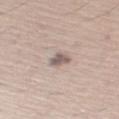Notes:
- follow-up · no biopsy performed (imaged during a skin exam)
- image · ~15 mm tile from a whole-body skin photo
- body site · the right upper arm
- lesion diameter · about 2.5 mm
- automated lesion analysis · a border-irregularity index near 3/10, internal color variation of about 3 on a 0–10 scale, and radial color variation of about 1; a classifier nevus-likeness of about 0/100 and a detector confidence of about 75 out of 100 that the crop contains a lesion
- lighting · white-light
- subject · male, roughly 60 years of age